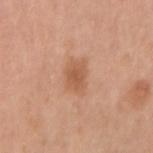{"biopsy_status": "not biopsied; imaged during a skin examination", "lighting": "white-light", "image": {"source": "total-body photography crop", "field_of_view_mm": 15}, "automated_metrics": {"nevus_likeness_0_100": 70, "lesion_detection_confidence_0_100": 100}, "lesion_size": {"long_diameter_mm_approx": 3.0}, "site": "right upper arm", "patient": {"sex": "female", "age_approx": 50}}